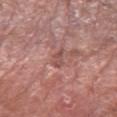{"biopsy_status": "not biopsied; imaged during a skin examination", "patient": {"sex": "male", "age_approx": 80}, "lesion_size": {"long_diameter_mm_approx": 2.5}, "site": "left forearm", "image": {"source": "total-body photography crop", "field_of_view_mm": 15}, "automated_metrics": {"cielab_L": 50, "cielab_a": 24, "cielab_b": 23, "vs_skin_darker_L": 9.0, "vs_skin_contrast_norm": 6.0, "nevus_likeness_0_100": 0, "lesion_detection_confidence_0_100": 55}}